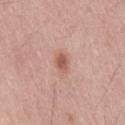Captured during whole-body skin photography for melanoma surveillance; the lesion was not biopsied. A male subject, aged approximately 55. Cropped from a total-body skin-imaging series; the visible field is about 15 mm. Located on the leg. The lesion's longest dimension is about 2.5 mm. The tile uses white-light illumination.